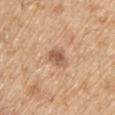{
  "biopsy_status": "not biopsied; imaged during a skin examination",
  "lesion_size": {
    "long_diameter_mm_approx": 2.5
  },
  "lighting": "white-light",
  "site": "upper back",
  "patient": {
    "sex": "male",
    "age_approx": 70
  },
  "image": {
    "source": "total-body photography crop",
    "field_of_view_mm": 15
  }
}